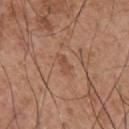Assessment: Imaged during a routine full-body skin examination; the lesion was not biopsied and no histopathology is available. Image and clinical context: Imaged with white-light lighting. Located on the chest. The subject is a male aged approximately 55. Automated tile analysis of the lesion measured an area of roughly 3 mm², an eccentricity of roughly 0.85, and a symmetry-axis asymmetry near 0.25. The analysis additionally found a lesion color around L≈50 a*≈22 b*≈31 in CIELAB, roughly 6 lightness units darker than nearby skin, and a normalized lesion–skin contrast near 5. A 15 mm close-up tile from a total-body photography series done for melanoma screening. Approximately 2.5 mm at its widest.